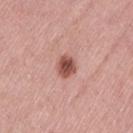Part of a total-body skin-imaging series; this lesion was reviewed on a skin check and was not flagged for biopsy. From the lower back. The patient is a female aged 38–42. Imaged with white-light lighting. A roughly 15 mm field-of-view crop from a total-body skin photograph. Measured at roughly 2.5 mm in maximum diameter.